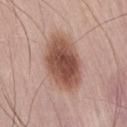Impression: Part of a total-body skin-imaging series; this lesion was reviewed on a skin check and was not flagged for biopsy. Context: Located on the lower back. A 15 mm close-up tile from a total-body photography series done for melanoma screening. Automated tile analysis of the lesion measured an automated nevus-likeness rating near 95 out of 100 and a lesion-detection confidence of about 100/100. Longest diameter approximately 6.5 mm. This is a white-light tile. The subject is a male about 60 years old.